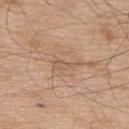The lesion was photographed on a routine skin check and not biopsied; there is no pathology result. A male patient, in their mid-70s. A 15 mm close-up extracted from a 3D total-body photography capture. Located on the back.